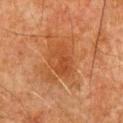Findings:
- biopsy status — imaged on a skin check; not biopsied
- site — the abdomen
- tile lighting — cross-polarized illumination
- image source — 15 mm crop, total-body photography
- automated lesion analysis — an outline eccentricity of about 0.7 (0 = round, 1 = elongated); a border-irregularity rating of about 2.5/10 and peripheral color asymmetry of about 1
- subject — male, aged 78 to 82